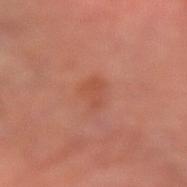This lesion was catalogued during total-body skin photography and was not selected for biopsy.
This image is a 15 mm lesion crop taken from a total-body photograph.
This is a cross-polarized tile.
From the arm.
A male patient, in their 70s.